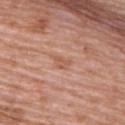Q: Was a biopsy performed?
A: imaged on a skin check; not biopsied
Q: What are the patient's age and sex?
A: female, about 70 years old
Q: How was this image acquired?
A: 15 mm crop, total-body photography
Q: What lighting was used for the tile?
A: white-light illumination
Q: What is the anatomic site?
A: the upper back